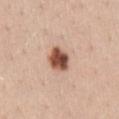Impression: Captured during whole-body skin photography for melanoma surveillance; the lesion was not biopsied. Context: The patient is a female about 40 years old. A roughly 15 mm field-of-view crop from a total-body skin photograph. On the back.